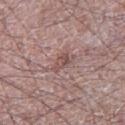| field | value |
|---|---|
| biopsy status | imaged on a skin check; not biopsied |
| illumination | white-light illumination |
| site | the right thigh |
| automated lesion analysis | an automated nevus-likeness rating near 0 out of 100 |
| subject | male, in their mid- to late 60s |
| lesion diameter | ≈2.5 mm |
| image | ~15 mm crop, total-body skin-cancer survey |